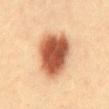notes = total-body-photography surveillance lesion; no biopsy | image source = 15 mm crop, total-body photography | patient = male, approximately 40 years of age | location = the mid back.